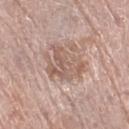workup: catalogued during a skin exam; not biopsied | tile lighting: white-light illumination | body site: the leg | image: 15 mm crop, total-body photography | lesion diameter: ≈5.5 mm | subject: female, aged 63 to 67.